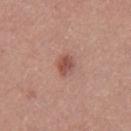The lesion was tiled from a total-body skin photograph and was not biopsied. Automated image analysis of the tile measured a lesion area of about 3.5 mm² and a symmetry-axis asymmetry near 0.25. It also reported roughly 11 lightness units darker than nearby skin and a normalized border contrast of about 8. The software also gave a nevus-likeness score of about 90/100 and a detector confidence of about 100 out of 100 that the crop contains a lesion. This is a white-light tile. A female patient approximately 35 years of age. Measured at roughly 2.5 mm in maximum diameter. A roughly 15 mm field-of-view crop from a total-body skin photograph. The lesion is on the left upper arm.